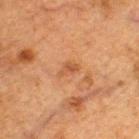automated metrics: a lesion color around L≈43 a*≈21 b*≈32 in CIELAB, a lesion–skin lightness drop of about 7, and a normalized border contrast of about 5.5; a border-irregularity index near 3/10, a color-variation rating of about 1.5/10, and peripheral color asymmetry of about 0.5 | lighting: cross-polarized illumination | subject: male, aged approximately 50 | image source: ~15 mm crop, total-body skin-cancer survey | size: ≈2.5 mm | location: the upper back.